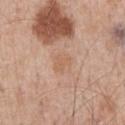{"biopsy_status": "not biopsied; imaged during a skin examination", "patient": {"sex": "male", "age_approx": 55}, "site": "chest", "image": {"source": "total-body photography crop", "field_of_view_mm": 15}}